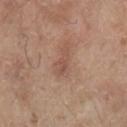The lesion was photographed on a routine skin check and not biopsied; there is no pathology result. The tile uses white-light illumination. Automated tile analysis of the lesion measured a lesion area of about 3 mm² and a shape eccentricity near 0.9. And it measured about 7 CIELAB-L* units darker than the surrounding skin and a normalized lesion–skin contrast near 5.5. It also reported an automated nevus-likeness rating near 0 out of 100 and lesion-presence confidence of about 100/100. A male patient, aged 78 to 82. A roughly 15 mm field-of-view crop from a total-body skin photograph. The recorded lesion diameter is about 3 mm. The lesion is on the right lower leg.